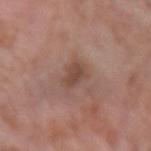Impression:
No biopsy was performed on this lesion — it was imaged during a full skin examination and was not determined to be concerning.
Image and clinical context:
The lesion is located on the left forearm. Imaged with white-light lighting. A female patient aged around 60. The lesion-visualizer software estimated an area of roughly 6 mm², an outline eccentricity of about 0.7 (0 = round, 1 = elongated), and a symmetry-axis asymmetry near 0.3. The software also gave a classifier nevus-likeness of about 5/100. A 15 mm close-up extracted from a 3D total-body photography capture. The lesion's longest dimension is about 3 mm.